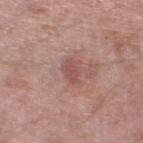Assessment:
No biopsy was performed on this lesion — it was imaged during a full skin examination and was not determined to be concerning.
Background:
The subject is a male aged 63–67. Imaged with white-light lighting. Cropped from a whole-body photographic skin survey; the tile spans about 15 mm. The lesion is on the left lower leg. About 3 mm across.